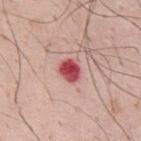| feature | finding |
|---|---|
| follow-up | catalogued during a skin exam; not biopsied |
| imaging modality | ~15 mm crop, total-body skin-cancer survey |
| subject | male, in their mid-30s |
| body site | the mid back |